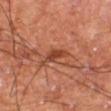No biopsy was performed on this lesion — it was imaged during a full skin examination and was not determined to be concerning. The total-body-photography lesion software estimated a color-variation rating of about 0.5/10 and peripheral color asymmetry of about 0. The patient is a male aged 58–62. The lesion is on the right thigh. Imaged with cross-polarized lighting. Cropped from a total-body skin-imaging series; the visible field is about 15 mm.